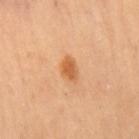The lesion was tiled from a total-body skin photograph and was not biopsied.